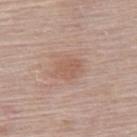This lesion was catalogued during total-body skin photography and was not selected for biopsy. Imaged with white-light lighting. Located on the upper back. This image is a 15 mm lesion crop taken from a total-body photograph. A female patient, aged around 65.